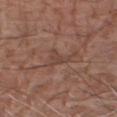Captured during whole-body skin photography for melanoma surveillance; the lesion was not biopsied. Longest diameter approximately 2.5 mm. The patient is a male in their 80s. The lesion is located on the right forearm. Cropped from a whole-body photographic skin survey; the tile spans about 15 mm.